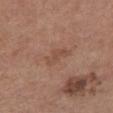| feature | finding |
|---|---|
| follow-up | total-body-photography surveillance lesion; no biopsy |
| illumination | white-light |
| body site | the abdomen |
| patient | female, roughly 65 years of age |
| diameter | ~3.5 mm (longest diameter) |
| image-analysis metrics | an area of roughly 4.5 mm² and a shape-asymmetry score of about 0.4 (0 = symmetric); an average lesion color of about L≈49 a*≈20 b*≈28 (CIELAB), a lesion–skin lightness drop of about 6, and a lesion-to-skin contrast of about 5 (normalized; higher = more distinct); border irregularity of about 4.5 on a 0–10 scale and a within-lesion color-variation index near 1/10; a lesion-detection confidence of about 100/100 |
| acquisition | ~15 mm tile from a whole-body skin photo |